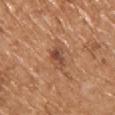Notes:
– notes — total-body-photography surveillance lesion; no biopsy
– image — total-body-photography crop, ~15 mm field of view
– patient — male, aged 68–72
– site — the upper back
– lesion size — about 3 mm
– illumination — white-light